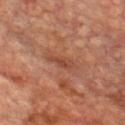Findings:
– notes · catalogued during a skin exam; not biopsied
– image-analysis metrics · an area of roughly 4 mm², an eccentricity of roughly 0.95, and a shape-asymmetry score of about 0.3 (0 = symmetric); a nevus-likeness score of about 0/100 and a detector confidence of about 65 out of 100 that the crop contains a lesion
– subject · male, aged around 75
– size · about 3.5 mm
– image · total-body-photography crop, ~15 mm field of view
– body site · the chest